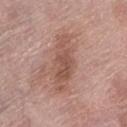A 15 mm close-up tile from a total-body photography series done for melanoma screening.
Located on the left lower leg.
About 6.5 mm across.
Automated tile analysis of the lesion measured a footprint of about 12 mm² and a shape eccentricity near 0.95. The analysis additionally found roughly 10 lightness units darker than nearby skin and a lesion-to-skin contrast of about 7.5 (normalized; higher = more distinct). The analysis additionally found a border-irregularity index near 5/10, a color-variation rating of about 3/10, and peripheral color asymmetry of about 1.
Captured under white-light illumination.
The subject is a female aged around 60.